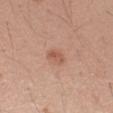notes: total-body-photography surveillance lesion; no biopsy | image-analysis metrics: a footprint of about 3 mm² and a shape-asymmetry score of about 0.25 (0 = symmetric); an average lesion color of about L≈56 a*≈24 b*≈31 (CIELAB), about 9 CIELAB-L* units darker than the surrounding skin, and a normalized lesion–skin contrast near 6.5; a border-irregularity rating of about 2.5/10, a color-variation rating of about 2.5/10, and peripheral color asymmetry of about 1; a classifier nevus-likeness of about 45/100 and a lesion-detection confidence of about 100/100 | site: the arm | illumination: white-light | imaging modality: 15 mm crop, total-body photography | diameter: about 2.5 mm | subject: female, in their mid-20s.